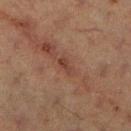Context: Automated tile analysis of the lesion measured an area of roughly 3 mm², an outline eccentricity of about 0.9 (0 = round, 1 = elongated), and two-axis asymmetry of about 0.35. The analysis additionally found a lesion color around L≈33 a*≈19 b*≈23 in CIELAB, a lesion–skin lightness drop of about 6, and a normalized lesion–skin contrast near 6.5. It also reported a border-irregularity index near 3.5/10, a within-lesion color-variation index near 0.5/10, and a peripheral color-asymmetry measure near 0. The software also gave lesion-presence confidence of about 100/100. The recorded lesion diameter is about 2.5 mm. The tile uses cross-polarized illumination. A 15 mm close-up extracted from a 3D total-body photography capture. A female subject, aged approximately 40. Located on the right lower leg.